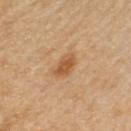  biopsy_status: not biopsied; imaged during a skin examination